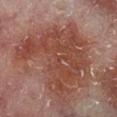Findings:
* biopsy status: total-body-photography surveillance lesion; no biopsy
* image-analysis metrics: roughly 7 lightness units darker than nearby skin and a normalized lesion–skin contrast near 7.5; a within-lesion color-variation index near 4/10 and peripheral color asymmetry of about 1
* lighting: cross-polarized illumination
* anatomic site: the right lower leg
* image: ~15 mm crop, total-body skin-cancer survey
* patient: male, in their 60s